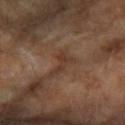Impression:
The lesion was photographed on a routine skin check and not biopsied; there is no pathology result.
Clinical summary:
This is a cross-polarized tile. On the right forearm. The patient is a female approximately 70 years of age. Cropped from a total-body skin-imaging series; the visible field is about 15 mm. Measured at roughly 3 mm in maximum diameter. The total-body-photography lesion software estimated a mean CIELAB color near L≈36 a*≈18 b*≈27, about 7 CIELAB-L* units darker than the surrounding skin, and a lesion-to-skin contrast of about 6 (normalized; higher = more distinct). The software also gave a classifier nevus-likeness of about 0/100 and a detector confidence of about 80 out of 100 that the crop contains a lesion.